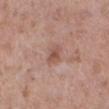Clinical impression: Recorded during total-body skin imaging; not selected for excision or biopsy. Clinical summary: The lesion-visualizer software estimated a peripheral color-asymmetry measure near 1. The software also gave a classifier nevus-likeness of about 0/100. A female patient, aged around 50. The lesion is located on the leg. The tile uses white-light illumination. Longest diameter approximately 2.5 mm. A region of skin cropped from a whole-body photographic capture, roughly 15 mm wide.